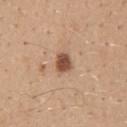| field | value |
|---|---|
| biopsy status | no biopsy performed (imaged during a skin exam) |
| patient | male, aged 38–42 |
| image | ~15 mm crop, total-body skin-cancer survey |
| image-analysis metrics | a mean CIELAB color near L≈51 a*≈21 b*≈31 and a normalized lesion–skin contrast near 10.5; a border-irregularity index near 1.5/10, a within-lesion color-variation index near 3/10, and a peripheral color-asymmetry measure near 1 |
| anatomic site | the mid back |
| illumination | white-light |